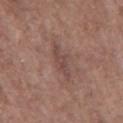{
  "biopsy_status": "not biopsied; imaged during a skin examination",
  "patient": {
    "sex": "male",
    "age_approx": 70
  },
  "image": {
    "source": "total-body photography crop",
    "field_of_view_mm": 15
  },
  "automated_metrics": {
    "border_irregularity_0_10": 5.5,
    "color_variation_0_10": 1.5,
    "peripheral_color_asymmetry": 0.5
  },
  "lighting": "white-light",
  "site": "mid back",
  "lesion_size": {
    "long_diameter_mm_approx": 4.0
  }
}